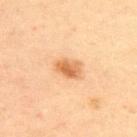Q: Was this lesion biopsied?
A: catalogued during a skin exam; not biopsied
Q: Lesion size?
A: ~3.5 mm (longest diameter)
Q: Where on the body is the lesion?
A: the upper back
Q: What lighting was used for the tile?
A: cross-polarized
Q: What kind of image is this?
A: ~15 mm tile from a whole-body skin photo
Q: What are the patient's age and sex?
A: male, approximately 45 years of age